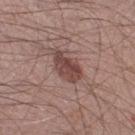Q: Was a biopsy performed?
A: imaged on a skin check; not biopsied
Q: What is the lesion's diameter?
A: about 4.5 mm
Q: Patient demographics?
A: male, aged around 55
Q: What lighting was used for the tile?
A: white-light
Q: What is the anatomic site?
A: the right lower leg
Q: What kind of image is this?
A: ~15 mm crop, total-body skin-cancer survey